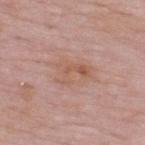Impression:
Recorded during total-body skin imaging; not selected for excision or biopsy.
Acquisition and patient details:
This is a white-light tile. A 15 mm crop from a total-body photograph taken for skin-cancer surveillance. The subject is a male in their 50s. Approximately 4 mm at its widest. The lesion is on the upper back. Automated image analysis of the tile measured a footprint of about 6 mm², a shape eccentricity near 0.8, and a shape-asymmetry score of about 0.6 (0 = symmetric). The software also gave a color-variation rating of about 2.5/10 and peripheral color asymmetry of about 0.